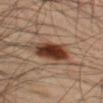{"biopsy_status": "not biopsied; imaged during a skin examination", "image": {"source": "total-body photography crop", "field_of_view_mm": 15}, "patient": {"sex": "male", "age_approx": 45}, "site": "left thigh"}